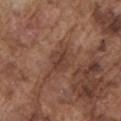Part of a total-body skin-imaging series; this lesion was reviewed on a skin check and was not flagged for biopsy.
Imaged with white-light lighting.
Approximately 2.5 mm at its widest.
From the left upper arm.
A male subject, about 75 years old.
The lesion-visualizer software estimated an area of roughly 5.5 mm² and a shape eccentricity near 0.65. The software also gave an automated nevus-likeness rating near 5 out of 100 and a lesion-detection confidence of about 95/100.
This image is a 15 mm lesion crop taken from a total-body photograph.